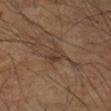workup: imaged on a skin check; not biopsied
anatomic site: the arm
image: 15 mm crop, total-body photography
patient: male, approximately 65 years of age